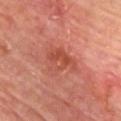  biopsy_status: not biopsied; imaged during a skin examination